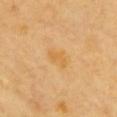No biopsy was performed on this lesion — it was imaged during a full skin examination and was not determined to be concerning. The lesion is located on the chest. A male subject, roughly 60 years of age. Automated tile analysis of the lesion measured an area of roughly 4.5 mm². And it measured a lesion color around L≈63 a*≈19 b*≈47 in CIELAB and a normalized lesion–skin contrast near 5.5. And it measured radial color variation of about 0.5. The software also gave a nevus-likeness score of about 0/100. A region of skin cropped from a whole-body photographic capture, roughly 15 mm wide.